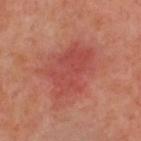| field | value |
|---|---|
| acquisition | ~15 mm tile from a whole-body skin photo |
| site | the upper back |
| lighting | cross-polarized illumination |
| subject | male, about 50 years old |
| lesion diameter | about 7 mm |
| image-analysis metrics | roughly 7 lightness units darker than nearby skin and a normalized border contrast of about 5.5; a border-irregularity index near 4/10 and a within-lesion color-variation index near 3/10; an automated nevus-likeness rating near 0 out of 100 |